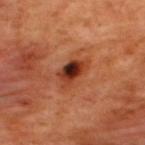An algorithmic analysis of the crop reported a lesion area of about 6.5 mm², a shape eccentricity near 0.8, and a symmetry-axis asymmetry near 0.2. The analysis additionally found a mean CIELAB color near L≈36 a*≈29 b*≈34. The lesion is located on the upper back. A male subject, roughly 50 years of age. About 4 mm across. This image is a 15 mm lesion crop taken from a total-body photograph.